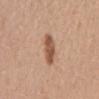The lesion was tiled from a total-body skin photograph and was not biopsied.
Longest diameter approximately 4 mm.
The tile uses white-light illumination.
The patient is a female aged 53–57.
The lesion-visualizer software estimated an area of roughly 6.5 mm². The software also gave a lesion color around L≈54 a*≈22 b*≈31 in CIELAB, about 13 CIELAB-L* units darker than the surrounding skin, and a lesion-to-skin contrast of about 9 (normalized; higher = more distinct). And it measured a classifier nevus-likeness of about 95/100 and a lesion-detection confidence of about 100/100.
A 15 mm close-up extracted from a 3D total-body photography capture.
Located on the mid back.